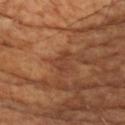Part of a total-body skin-imaging series; this lesion was reviewed on a skin check and was not flagged for biopsy.